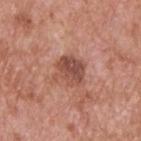Q: What are the patient's age and sex?
A: male, aged approximately 60
Q: What is the lesion's diameter?
A: ≈3.5 mm
Q: What is the imaging modality?
A: 15 mm crop, total-body photography
Q: Automated lesion metrics?
A: a footprint of about 9 mm², a shape eccentricity near 0.45, and two-axis asymmetry of about 0.25
Q: Where on the body is the lesion?
A: the upper back
Q: How was the tile lit?
A: white-light illumination